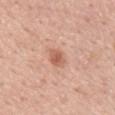workup: imaged on a skin check; not biopsied | lighting: white-light | diameter: about 2.5 mm | patient: male, aged around 55 | image source: total-body-photography crop, ~15 mm field of view | site: the mid back | image-analysis metrics: a lesion area of about 4.5 mm², a shape eccentricity near 0.5, and two-axis asymmetry of about 0.2; a border-irregularity rating of about 1.5/10, internal color variation of about 2.5 on a 0–10 scale, and a peripheral color-asymmetry measure near 0.5; a nevus-likeness score of about 85/100.